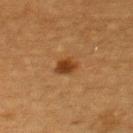An algorithmic analysis of the crop reported roughly 10 lightness units darker than nearby skin and a normalized border contrast of about 9. The software also gave internal color variation of about 2.5 on a 0–10 scale and a peripheral color-asymmetry measure near 1. A 15 mm close-up extracted from a 3D total-body photography capture. From the chest. The tile uses cross-polarized illumination. The lesion's longest dimension is about 2.5 mm. The patient is a female about 55 years old.